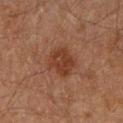Notes:
* notes: imaged on a skin check; not biopsied
* diameter: ≈3.5 mm
* anatomic site: the leg
* patient: male, roughly 60 years of age
* image: ~15 mm tile from a whole-body skin photo
* lighting: cross-polarized